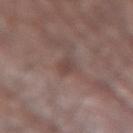anatomic site: the left forearm
patient: male, roughly 80 years of age
image: ~15 mm tile from a whole-body skin photo
automated metrics: an average lesion color of about L≈43 a*≈17 b*≈20 (CIELAB), roughly 7 lightness units darker than nearby skin, and a normalized lesion–skin contrast near 5.5; an automated nevus-likeness rating near 0 out of 100
tile lighting: white-light